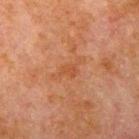| key | value |
|---|---|
| follow-up | imaged on a skin check; not biopsied |
| site | the left upper arm |
| automated lesion analysis | border irregularity of about 6.5 on a 0–10 scale, a within-lesion color-variation index near 1/10, and radial color variation of about 0.5 |
| acquisition | total-body-photography crop, ~15 mm field of view |
| patient | male, aged around 80 |
| lighting | cross-polarized illumination |
| lesion size | about 4.5 mm |